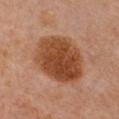workup — catalogued during a skin exam; not biopsied
subject — female, approximately 60 years of age
TBP lesion metrics — a footprint of about 30 mm² and an outline eccentricity of about 0.7 (0 = round, 1 = elongated)
image — 15 mm crop, total-body photography
location — the chest
lighting — cross-polarized illumination
lesion diameter — ~7.5 mm (longest diameter)